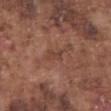<case>
  <biopsy_status>not biopsied; imaged during a skin examination</biopsy_status>
  <automated_metrics>
    <shape_asymmetry>0.4</shape_asymmetry>
    <border_irregularity_0_10>4.0</border_irregularity_0_10>
    <color_variation_0_10>0.5</color_variation_0_10>
    <peripheral_color_asymmetry>0.0</peripheral_color_asymmetry>
  </automated_metrics>
  <patient>
    <sex>male</sex>
    <age_approx>75</age_approx>
  </patient>
  <image>
    <source>total-body photography crop</source>
    <field_of_view_mm>15</field_of_view_mm>
  </image>
  <lighting>white-light</lighting>
  <lesion_size>
    <long_diameter_mm_approx>2.5</long_diameter_mm_approx>
  </lesion_size>
  <site>abdomen</site>
</case>